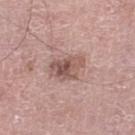{"biopsy_status": "not biopsied; imaged during a skin examination", "site": "right lower leg", "automated_metrics": {"border_irregularity_0_10": 3.0, "color_variation_0_10": 6.5, "peripheral_color_asymmetry": 2.5, "nevus_likeness_0_100": 30, "lesion_detection_confidence_0_100": 100}, "patient": {"sex": "male", "age_approx": 75}, "lesion_size": {"long_diameter_mm_approx": 4.0}, "image": {"source": "total-body photography crop", "field_of_view_mm": 15}}